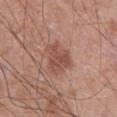Context: Automated image analysis of the tile measured a mean CIELAB color near L≈50 a*≈23 b*≈27, roughly 9 lightness units darker than nearby skin, and a normalized border contrast of about 6.5. The software also gave a within-lesion color-variation index near 3/10 and a peripheral color-asymmetry measure near 1. A male patient, aged 68–72. Cropped from a whole-body photographic skin survey; the tile spans about 15 mm. From the abdomen. The lesion's longest dimension is about 3.5 mm.